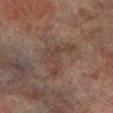<case>
  <biopsy_status>not biopsied; imaged during a skin examination</biopsy_status>
  <site>leg</site>
  <lesion_size>
    <long_diameter_mm_approx>5.5</long_diameter_mm_approx>
  </lesion_size>
  <patient>
    <sex>male</sex>
    <age_approx>75</age_approx>
  </patient>
  <automated_metrics>
    <vs_skin_darker_L>5.0</vs_skin_darker_L>
    <vs_skin_contrast_norm>5.5</vs_skin_contrast_norm>
    <border_irregularity_0_10>10.0</border_irregularity_0_10>
    <color_variation_0_10>1.0</color_variation_0_10>
    <peripheral_color_asymmetry>0.5</peripheral_color_asymmetry>
  </automated_metrics>
  <image>
    <source>total-body photography crop</source>
    <field_of_view_mm>15</field_of_view_mm>
  </image>
</case>